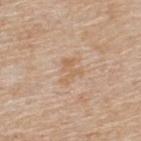Notes:
• biopsy status · total-body-photography surveillance lesion; no biopsy
• subject · male, aged around 55
• tile lighting · white-light illumination
• image source · ~15 mm crop, total-body skin-cancer survey
• site · the upper back
• TBP lesion metrics · a footprint of about 5 mm², an eccentricity of roughly 0.65, and a symmetry-axis asymmetry near 0.5
• diameter · ≈3 mm